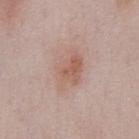Impression:
No biopsy was performed on this lesion — it was imaged during a full skin examination and was not determined to be concerning.
Clinical summary:
Automated tile analysis of the lesion measured an area of roughly 13 mm², an outline eccentricity of about 0.75 (0 = round, 1 = elongated), and a symmetry-axis asymmetry near 0.15. It also reported an automated nevus-likeness rating near 35 out of 100 and a detector confidence of about 100 out of 100 that the crop contains a lesion. Imaged with white-light lighting. Cropped from a whole-body photographic skin survey; the tile spans about 15 mm. The patient is a male about 55 years old. The lesion is on the chest.